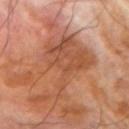<case>
<biopsy_status>not biopsied; imaged during a skin examination</biopsy_status>
<patient>
  <sex>male</sex>
  <age_approx>70</age_approx>
</patient>
<image>
  <source>total-body photography crop</source>
  <field_of_view_mm>15</field_of_view_mm>
</image>
<site>left forearm</site>
</case>